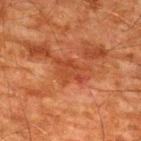biopsy status = imaged on a skin check; not biopsied
image source = ~15 mm crop, total-body skin-cancer survey
body site = the upper back
subject = male, about 60 years old